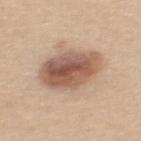Assessment: Part of a total-body skin-imaging series; this lesion was reviewed on a skin check and was not flagged for biopsy. Acquisition and patient details: A female subject, in their 40s. The lesion's longest dimension is about 6 mm. Automated tile analysis of the lesion measured a lesion color around L≈56 a*≈19 b*≈28 in CIELAB, roughly 15 lightness units darker than nearby skin, and a normalized lesion–skin contrast near 9.5. The analysis additionally found peripheral color asymmetry of about 2. Located on the mid back. Captured under white-light illumination. A 15 mm close-up extracted from a 3D total-body photography capture.